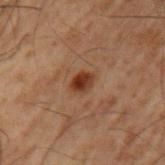follow-up: catalogued during a skin exam; not biopsied
patient: male, aged approximately 50
lesion size: about 2.5 mm
location: the left upper arm
lighting: cross-polarized illumination
imaging modality: ~15 mm tile from a whole-body skin photo
image-analysis metrics: a border-irregularity index near 2.5/10, internal color variation of about 4 on a 0–10 scale, and radial color variation of about 1